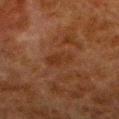Findings:
– notes · no biopsy performed (imaged during a skin exam)
– image-analysis metrics · a footprint of about 4.5 mm², an eccentricity of roughly 0.75, and a shape-asymmetry score of about 0.2 (0 = symmetric); a lesion color around L≈26 a*≈19 b*≈26 in CIELAB, about 4 CIELAB-L* units darker than the surrounding skin, and a lesion-to-skin contrast of about 5.5 (normalized; higher = more distinct); a classifier nevus-likeness of about 0/100
– patient · male, approximately 80 years of age
– size · ≈3 mm
– image · total-body-photography crop, ~15 mm field of view
– anatomic site · the left lower leg